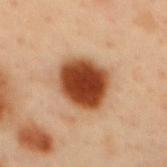Q: Was a biopsy performed?
A: total-body-photography surveillance lesion; no biopsy
Q: How large is the lesion?
A: ≈5 mm
Q: How was this image acquired?
A: ~15 mm tile from a whole-body skin photo
Q: What is the anatomic site?
A: the back
Q: How was the tile lit?
A: cross-polarized
Q: What did automated image analysis measure?
A: a mean CIELAB color near L≈46 a*≈28 b*≈38, roughly 22 lightness units darker than nearby skin, and a normalized border contrast of about 15; a nevus-likeness score of about 100/100 and a lesion-detection confidence of about 100/100
Q: Who is the patient?
A: male, approximately 40 years of age